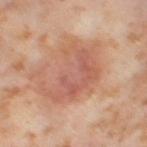{
  "biopsy_status": "not biopsied; imaged during a skin examination",
  "lighting": "cross-polarized",
  "lesion_size": {
    "long_diameter_mm_approx": 7.0
  },
  "site": "right thigh",
  "image": {
    "source": "total-body photography crop",
    "field_of_view_mm": 15
  },
  "patient": {
    "sex": "female",
    "age_approx": 55
  }
}